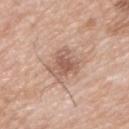<record>
  <biopsy_status>not biopsied; imaged during a skin examination</biopsy_status>
  <automated_metrics>
    <color_variation_0_10>4.0</color_variation_0_10>
    <peripheral_color_asymmetry>1.0</peripheral_color_asymmetry>
  </automated_metrics>
  <image>
    <source>total-body photography crop</source>
    <field_of_view_mm>15</field_of_view_mm>
  </image>
  <lighting>white-light</lighting>
  <patient>
    <sex>male</sex>
    <age_approx>65</age_approx>
  </patient>
  <site>upper back</site>
  <lesion_size>
    <long_diameter_mm_approx>4.0</long_diameter_mm_approx>
  </lesion_size>
</record>